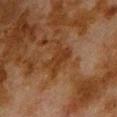Q: Was this lesion biopsied?
A: total-body-photography surveillance lesion; no biopsy
Q: Who is the patient?
A: male, approximately 80 years of age
Q: What did automated image analysis measure?
A: a lesion area of about 7.5 mm², an eccentricity of roughly 0.75, and two-axis asymmetry of about 0.35; a mean CIELAB color near L≈28 a*≈18 b*≈29 and a normalized lesion–skin contrast near 6.5; a border-irregularity rating of about 5.5/10, a color-variation rating of about 2/10, and peripheral color asymmetry of about 1
Q: What is the lesion's diameter?
A: ~4 mm (longest diameter)
Q: What is the imaging modality?
A: 15 mm crop, total-body photography
Q: How was the tile lit?
A: cross-polarized
Q: What is the anatomic site?
A: the upper back